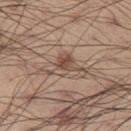biopsy_status: not biopsied; imaged during a skin examination
site: left thigh
image:
  source: total-body photography crop
  field_of_view_mm: 15
patient:
  sex: male
  age_approx: 55
lighting: white-light
automated_metrics:
  area_mm2_approx: 7.0
  eccentricity: 0.75